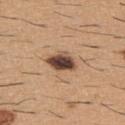The lesion was photographed on a routine skin check and not biopsied; there is no pathology result.
Located on the upper back.
Captured under white-light illumination.
An algorithmic analysis of the crop reported a lesion area of about 7 mm², an eccentricity of roughly 0.7, and two-axis asymmetry of about 0.2. And it measured a lesion color around L≈45 a*≈18 b*≈27 in CIELAB, a lesion–skin lightness drop of about 20, and a normalized border contrast of about 14. And it measured a border-irregularity index near 2/10 and peripheral color asymmetry of about 2.5.
A male patient, aged approximately 60.
A 15 mm close-up tile from a total-body photography series done for melanoma screening.
About 3.5 mm across.